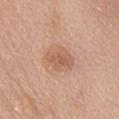Clinical summary: The lesion is on the abdomen. The subject is a female aged 58–62. A close-up tile cropped from a whole-body skin photograph, about 15 mm across. Captured under white-light illumination. The total-body-photography lesion software estimated an area of roughly 9.5 mm², a shape eccentricity near 0.65, and two-axis asymmetry of about 0.2. The analysis additionally found a mean CIELAB color near L≈60 a*≈21 b*≈32, a lesion–skin lightness drop of about 8, and a lesion-to-skin contrast of about 6 (normalized; higher = more distinct). It also reported a nevus-likeness score of about 20/100. Measured at roughly 4 mm in maximum diameter.